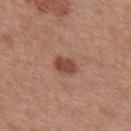Q: Was this lesion biopsied?
A: catalogued during a skin exam; not biopsied
Q: Who is the patient?
A: male, about 30 years old
Q: Automated lesion metrics?
A: a footprint of about 5 mm², a shape eccentricity near 0.7, and two-axis asymmetry of about 0.2; a mean CIELAB color near L≈46 a*≈23 b*≈29, about 11 CIELAB-L* units darker than the surrounding skin, and a lesion-to-skin contrast of about 8.5 (normalized; higher = more distinct); a border-irregularity rating of about 2/10, a color-variation rating of about 2/10, and radial color variation of about 0.5; a nevus-likeness score of about 75/100 and a lesion-detection confidence of about 100/100
Q: What is the anatomic site?
A: the upper back
Q: What kind of image is this?
A: ~15 mm tile from a whole-body skin photo